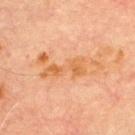{"biopsy_status": "not biopsied; imaged during a skin examination", "lesion_size": {"long_diameter_mm_approx": 7.5}, "patient": {"sex": "male", "age_approx": 70}, "automated_metrics": {"vs_skin_darker_L": 7.0, "vs_skin_contrast_norm": 6.5, "color_variation_0_10": 4.5, "peripheral_color_asymmetry": 1.5, "nevus_likeness_0_100": 0}, "site": "chest", "image": {"source": "total-body photography crop", "field_of_view_mm": 15}, "lighting": "cross-polarized"}